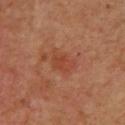Impression: This lesion was catalogued during total-body skin photography and was not selected for biopsy. Context: The lesion is on the upper back. A female patient aged 38–42. An algorithmic analysis of the crop reported an automated nevus-likeness rating near 10 out of 100 and lesion-presence confidence of about 100/100. This image is a 15 mm lesion crop taken from a total-body photograph.